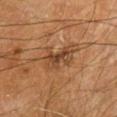{"biopsy_status": "not biopsied; imaged during a skin examination", "image": {"source": "total-body photography crop", "field_of_view_mm": 15}, "lesion_size": {"long_diameter_mm_approx": 3.5}, "site": "head or neck", "lighting": "cross-polarized", "patient": {"sex": "male", "age_approx": "85+"}, "automated_metrics": {"area_mm2_approx": 7.0, "eccentricity": 0.75, "shape_asymmetry": 0.35}}